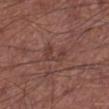<lesion>
  <biopsy_status>not biopsied; imaged during a skin examination</biopsy_status>
  <patient>
    <sex>male</sex>
    <age_approx>65</age_approx>
  </patient>
  <automated_metrics>
    <area_mm2_approx>4.5</area_mm2_approx>
    <eccentricity>0.8</eccentricity>
    <shape_asymmetry>0.55</shape_asymmetry>
    <cielab_L>39</cielab_L>
    <cielab_a>21</cielab_a>
    <cielab_b>23</cielab_b>
    <vs_skin_darker_L>6.0</vs_skin_darker_L>
    <vs_skin_contrast_norm>5.0</vs_skin_contrast_norm>
    <border_irregularity_0_10>7.0</border_irregularity_0_10>
    <color_variation_0_10>0.0</color_variation_0_10>
    <peripheral_color_asymmetry>0.0</peripheral_color_asymmetry>
    <nevus_likeness_0_100>0</nevus_likeness_0_100>
    <lesion_detection_confidence_0_100>100</lesion_detection_confidence_0_100>
  </automated_metrics>
  <image>
    <source>total-body photography crop</source>
    <field_of_view_mm>15</field_of_view_mm>
  </image>
  <site>left lower leg</site>
  <lesion_size>
    <long_diameter_mm_approx>3.0</long_diameter_mm_approx>
  </lesion_size>
</lesion>